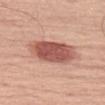follow-up = catalogued during a skin exam; not biopsied | lighting = white-light | patient = male, about 70 years old | image = total-body-photography crop, ~15 mm field of view | automated lesion analysis = a lesion area of about 18 mm², an eccentricity of roughly 0.8, and a symmetry-axis asymmetry near 0.15; a within-lesion color-variation index near 4.5/10 and peripheral color asymmetry of about 1 | size = ~6 mm (longest diameter) | body site = the left thigh.